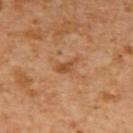Q: Is there a histopathology result?
A: total-body-photography surveillance lesion; no biopsy
Q: What lighting was used for the tile?
A: cross-polarized illumination
Q: How was this image acquired?
A: ~15 mm crop, total-body skin-cancer survey
Q: Automated lesion metrics?
A: a mean CIELAB color near L≈51 a*≈25 b*≈40 and a normalized border contrast of about 7
Q: Lesion location?
A: the upper back
Q: What are the patient's age and sex?
A: female, in their mid- to late 50s